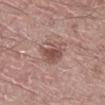Case summary:
• biopsy status · catalogued during a skin exam; not biopsied
• image · total-body-photography crop, ~15 mm field of view
• location · the right lower leg
• subject · male, in their 20s
• illumination · white-light illumination
• lesion size · ~3.5 mm (longest diameter)